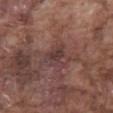The lesion was photographed on a routine skin check and not biopsied; there is no pathology result. On the abdomen. Automated image analysis of the tile measured a lesion–skin lightness drop of about 7 and a normalized lesion–skin contrast near 6.5. A male patient approximately 75 years of age. Cropped from a total-body skin-imaging series; the visible field is about 15 mm. The tile uses white-light illumination. The recorded lesion diameter is about 3 mm.